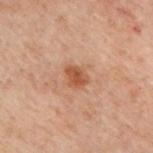Imaged during a routine full-body skin examination; the lesion was not biopsied and no histopathology is available. A 15 mm close-up tile from a total-body photography series done for melanoma screening. A male patient, aged around 50. The lesion is located on the back.